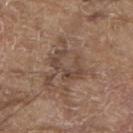Q: Was this lesion biopsied?
A: no biopsy performed (imaged during a skin exam)
Q: Where on the body is the lesion?
A: the upper back
Q: What kind of image is this?
A: ~15 mm tile from a whole-body skin photo
Q: How large is the lesion?
A: ~4.5 mm (longest diameter)
Q: What are the patient's age and sex?
A: male, aged 78–82
Q: Illumination type?
A: white-light